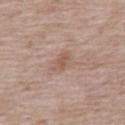{
  "biopsy_status": "not biopsied; imaged during a skin examination",
  "lighting": "white-light",
  "patient": {
    "sex": "male",
    "age_approx": 75
  },
  "image": {
    "source": "total-body photography crop",
    "field_of_view_mm": 15
  },
  "site": "back",
  "lesion_size": {
    "long_diameter_mm_approx": 3.0
  },
  "automated_metrics": {
    "area_mm2_approx": 4.0,
    "eccentricity": 0.8,
    "shape_asymmetry": 0.25,
    "color_variation_0_10": 1.5,
    "peripheral_color_asymmetry": 0.5,
    "lesion_detection_confidence_0_100": 100
  }
}